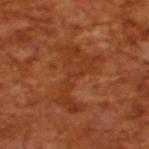| key | value |
|---|---|
| follow-up | catalogued during a skin exam; not biopsied |
| TBP lesion metrics | a border-irregularity rating of about 10/10, a color-variation rating of about 2/10, and radial color variation of about 0.5 |
| image source | ~15 mm crop, total-body skin-cancer survey |
| lesion size | about 7 mm |
| patient | male, about 65 years old |